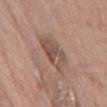Impression:
This lesion was catalogued during total-body skin photography and was not selected for biopsy.
Background:
A region of skin cropped from a whole-body photographic capture, roughly 15 mm wide. Longest diameter approximately 5 mm. Located on the abdomen. This is a white-light tile. The subject is a male about 70 years old.